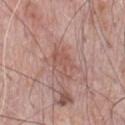The lesion was tiled from a total-body skin photograph and was not biopsied.
A 15 mm close-up tile from a total-body photography series done for melanoma screening.
A male subject aged 68 to 72.
On the chest.